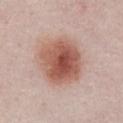Clinical impression:
Part of a total-body skin-imaging series; this lesion was reviewed on a skin check and was not flagged for biopsy.